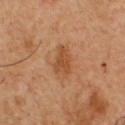- notes: total-body-photography surveillance lesion; no biopsy
- site: the chest
- imaging modality: total-body-photography crop, ~15 mm field of view
- subject: male, roughly 50 years of age